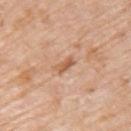Part of a total-body skin-imaging series; this lesion was reviewed on a skin check and was not flagged for biopsy. Cropped from a whole-body photographic skin survey; the tile spans about 15 mm. From the left upper arm. The patient is a female in their mid-70s. Captured under white-light illumination. About 2.5 mm across. The lesion-visualizer software estimated an area of roughly 3 mm² and an eccentricity of roughly 0.9. And it measured a mean CIELAB color near L≈59 a*≈22 b*≈35, a lesion–skin lightness drop of about 10, and a lesion-to-skin contrast of about 7 (normalized; higher = more distinct). It also reported a classifier nevus-likeness of about 0/100 and lesion-presence confidence of about 100/100.